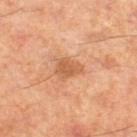body site = the right lower leg
acquisition = ~15 mm crop, total-body skin-cancer survey
subject = male, in their 60s
illumination = cross-polarized